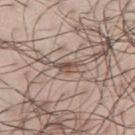No biopsy was performed on this lesion — it was imaged during a full skin examination and was not determined to be concerning. The lesion is located on the right upper arm. A male subject in their mid- to late 40s. Longest diameter approximately 3 mm. Captured under white-light illumination. A 15 mm close-up tile from a total-body photography series done for melanoma screening.